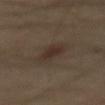Imaged during a routine full-body skin examination; the lesion was not biopsied and no histopathology is available. Measured at roughly 3.5 mm in maximum diameter. A 15 mm close-up tile from a total-body photography series done for melanoma screening. A male patient roughly 60 years of age. Captured under cross-polarized illumination. The lesion is on the mid back. The lesion-visualizer software estimated a shape eccentricity near 0.85 and a symmetry-axis asymmetry near 0.15. And it measured a border-irregularity index near 2/10, internal color variation of about 2.5 on a 0–10 scale, and peripheral color asymmetry of about 1.